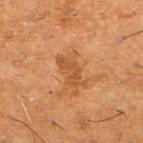Clinical impression:
No biopsy was performed on this lesion — it was imaged during a full skin examination and was not determined to be concerning.
Acquisition and patient details:
Cropped from a total-body skin-imaging series; the visible field is about 15 mm. Approximately 4 mm at its widest. An algorithmic analysis of the crop reported an eccentricity of roughly 0.85 and a symmetry-axis asymmetry near 0.45. The analysis additionally found border irregularity of about 5.5 on a 0–10 scale, a color-variation rating of about 2.5/10, and radial color variation of about 1. The software also gave lesion-presence confidence of about 100/100. A male patient, in their 60s. On the left lower leg. The tile uses cross-polarized illumination.